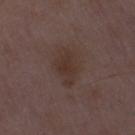biopsy status = imaged on a skin check; not biopsied | lesion diameter = ≈4.5 mm | illumination = white-light | image = ~15 mm crop, total-body skin-cancer survey | subject = male, approximately 50 years of age.